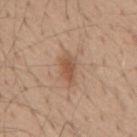Clinical impression:
The lesion was tiled from a total-body skin photograph and was not biopsied.
Clinical summary:
A male subject, aged 53–57. Captured under white-light illumination. Cropped from a total-body skin-imaging series; the visible field is about 15 mm. From the mid back. An algorithmic analysis of the crop reported border irregularity of about 3 on a 0–10 scale and radial color variation of about 1. The lesion's longest dimension is about 4 mm.